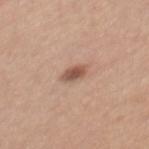Part of a total-body skin-imaging series; this lesion was reviewed on a skin check and was not flagged for biopsy. A female subject, approximately 35 years of age. An algorithmic analysis of the crop reported an average lesion color of about L≈54 a*≈20 b*≈27 (CIELAB) and a lesion-to-skin contrast of about 8.5 (normalized; higher = more distinct). It also reported a nevus-likeness score of about 85/100. Imaged with white-light lighting. A roughly 15 mm field-of-view crop from a total-body skin photograph. Located on the mid back. The lesion's longest dimension is about 3 mm.